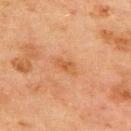Part of a total-body skin-imaging series; this lesion was reviewed on a skin check and was not flagged for biopsy. From the back. A close-up tile cropped from a whole-body skin photograph, about 15 mm across. Automated image analysis of the tile measured a footprint of about 4.5 mm², an eccentricity of roughly 0.85, and two-axis asymmetry of about 0.25. The software also gave border irregularity of about 3.5 on a 0–10 scale, internal color variation of about 2 on a 0–10 scale, and peripheral color asymmetry of about 0.5. And it measured an automated nevus-likeness rating near 0 out of 100 and a detector confidence of about 100 out of 100 that the crop contains a lesion. Captured under cross-polarized illumination. A male subject aged approximately 70. The recorded lesion diameter is about 3.5 mm.